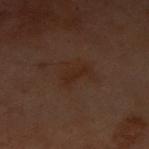Recorded during total-body skin imaging; not selected for excision or biopsy.
A female subject aged approximately 60.
An algorithmic analysis of the crop reported a lesion area of about 3 mm², an outline eccentricity of about 0.9 (0 = round, 1 = elongated), and a shape-asymmetry score of about 0.3 (0 = symmetric). The software also gave a lesion color around L≈20 a*≈16 b*≈22 in CIELAB, roughly 4 lightness units darker than nearby skin, and a lesion-to-skin contrast of about 6 (normalized; higher = more distinct). The analysis additionally found border irregularity of about 3.5 on a 0–10 scale, a within-lesion color-variation index near 0/10, and peripheral color asymmetry of about 0. The analysis additionally found a nevus-likeness score of about 0/100.
Approximately 3 mm at its widest.
From the front of the torso.
A 15 mm crop from a total-body photograph taken for skin-cancer surveillance.
The tile uses cross-polarized illumination.